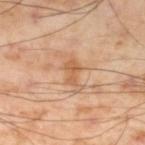biopsy status — catalogued during a skin exam; not biopsied
image source — 15 mm crop, total-body photography
size — ≈3 mm
illumination — cross-polarized illumination
anatomic site — the left lower leg
automated lesion analysis — an automated nevus-likeness rating near 0 out of 100 and a detector confidence of about 100 out of 100 that the crop contains a lesion
subject — male, in their mid-50s